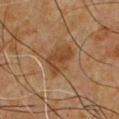Q: Was a biopsy performed?
A: catalogued during a skin exam; not biopsied
Q: Who is the patient?
A: male, aged approximately 60
Q: What did automated image analysis measure?
A: an area of roughly 10 mm², an eccentricity of roughly 0.7, and a symmetry-axis asymmetry near 0.25; border irregularity of about 2.5 on a 0–10 scale and peripheral color asymmetry of about 1
Q: How large is the lesion?
A: ≈4.5 mm
Q: Lesion location?
A: the chest
Q: What is the imaging modality?
A: ~15 mm crop, total-body skin-cancer survey